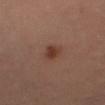Approximately 2.5 mm at its widest.
A roughly 15 mm field-of-view crop from a total-body skin photograph.
A male patient, aged 63 to 67.
Captured under cross-polarized illumination.
On the right thigh.